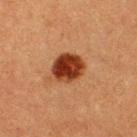biopsy status: total-body-photography surveillance lesion; no biopsy | patient: male, approximately 40 years of age | TBP lesion metrics: an area of roughly 10 mm²; a lesion–skin lightness drop of about 16 and a lesion-to-skin contrast of about 14 (normalized; higher = more distinct) | acquisition: ~15 mm tile from a whole-body skin photo | lighting: cross-polarized illumination | size: about 4 mm | location: the upper back.